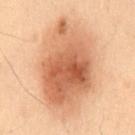Clinical impression:
This lesion was catalogued during total-body skin photography and was not selected for biopsy.
Clinical summary:
The patient is a male approximately 55 years of age. From the mid back. An algorithmic analysis of the crop reported an eccentricity of roughly 0.8 and a shape-asymmetry score of about 0.15 (0 = symmetric). The software also gave a nevus-likeness score of about 95/100 and lesion-presence confidence of about 100/100. Cropped from a whole-body photographic skin survey; the tile spans about 15 mm. The tile uses cross-polarized illumination. Measured at roughly 11.5 mm in maximum diameter.